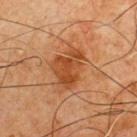The lesion was tiled from a total-body skin photograph and was not biopsied.
A male subject approximately 65 years of age.
Imaged with cross-polarized lighting.
Approximately 5 mm at its widest.
The lesion is on the chest.
A 15 mm close-up tile from a total-body photography series done for melanoma screening.